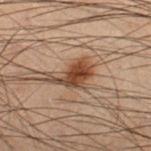A roughly 15 mm field-of-view crop from a total-body skin photograph. The lesion is on the leg. The subject is a male aged around 55.